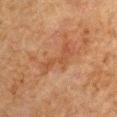Case summary:
* biopsy status — imaged on a skin check; not biopsied
* lesion size — about 5 mm
* body site — the left arm
* image source — total-body-photography crop, ~15 mm field of view
* patient — male, aged approximately 60